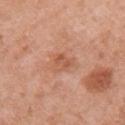Recorded during total-body skin imaging; not selected for excision or biopsy. A 15 mm close-up extracted from a 3D total-body photography capture. The patient is a female roughly 50 years of age. Located on the arm.